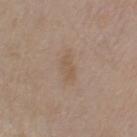site: left upper arm
patient:
  sex: male
  age_approx: 70
image:
  source: total-body photography crop
  field_of_view_mm: 15